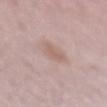biopsy status = imaged on a skin check; not biopsied
site = the arm
lighting = white-light illumination
acquisition = total-body-photography crop, ~15 mm field of view
patient = female, aged approximately 50